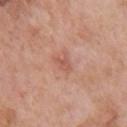Assessment:
Part of a total-body skin-imaging series; this lesion was reviewed on a skin check and was not flagged for biopsy.
Acquisition and patient details:
The total-body-photography lesion software estimated about 7 CIELAB-L* units darker than the surrounding skin and a normalized lesion–skin contrast near 5. It also reported a border-irregularity rating of about 3.5/10 and a within-lesion color-variation index near 2/10. And it measured an automated nevus-likeness rating near 0 out of 100 and a detector confidence of about 100 out of 100 that the crop contains a lesion. Imaged with white-light lighting. A male patient aged around 70. Cropped from a whole-body photographic skin survey; the tile spans about 15 mm. Located on the front of the torso. The recorded lesion diameter is about 2.5 mm.